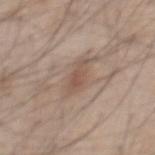<lesion>
<biopsy_status>not biopsied; imaged during a skin examination</biopsy_status>
<site>mid back</site>
<automated_metrics>
  <cielab_L>53</cielab_L>
  <cielab_a>16</cielab_a>
  <cielab_b>26</cielab_b>
  <vs_skin_darker_L>8.0</vs_skin_darker_L>
  <vs_skin_contrast_norm>6.0</vs_skin_contrast_norm>
</automated_metrics>
<image>
  <source>total-body photography crop</source>
  <field_of_view_mm>15</field_of_view_mm>
</image>
<patient>
  <sex>male</sex>
  <age_approx>45</age_approx>
</patient>
</lesion>